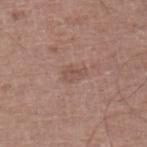Notes:
* biopsy status · no biopsy performed (imaged during a skin exam)